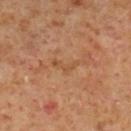Clinical impression:
Captured during whole-body skin photography for melanoma surveillance; the lesion was not biopsied.
Context:
Imaged with cross-polarized lighting. Approximately 3.5 mm at its widest. The lesion is on the right lower leg. A male patient about 60 years old. A 15 mm close-up extracted from a 3D total-body photography capture.